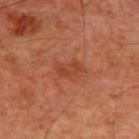Q: Is there a histopathology result?
A: catalogued during a skin exam; not biopsied
Q: What kind of image is this?
A: ~15 mm tile from a whole-body skin photo
Q: What are the patient's age and sex?
A: male, aged around 60
Q: How was the tile lit?
A: cross-polarized
Q: Where on the body is the lesion?
A: the chest
Q: How large is the lesion?
A: about 3.5 mm